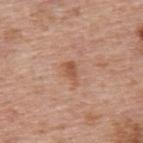The lesion was tiled from a total-body skin photograph and was not biopsied. Measured at roughly 2.5 mm in maximum diameter. Automated tile analysis of the lesion measured a lesion color around L≈54 a*≈23 b*≈33 in CIELAB, roughly 9 lightness units darker than nearby skin, and a normalized border contrast of about 7. The analysis additionally found border irregularity of about 4 on a 0–10 scale, internal color variation of about 1.5 on a 0–10 scale, and radial color variation of about 0.5. The analysis additionally found an automated nevus-likeness rating near 15 out of 100 and a lesion-detection confidence of about 100/100. Captured under white-light illumination. A male subject, about 75 years old. The lesion is on the upper back. A 15 mm crop from a total-body photograph taken for skin-cancer surveillance.